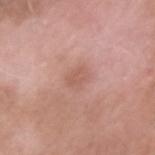biopsy status=imaged on a skin check; not biopsied
tile lighting=white-light
body site=the left upper arm
diameter=about 3 mm
image source=~15 mm crop, total-body skin-cancer survey
subject=male, in their 40s